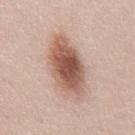<tbp_lesion>
  <biopsy_status>not biopsied; imaged during a skin examination</biopsy_status>
  <patient>
    <sex>male</sex>
    <age_approx>50</age_approx>
  </patient>
  <site>abdomen</site>
  <lesion_size>
    <long_diameter_mm_approx>8.5</long_diameter_mm_approx>
  </lesion_size>
  <image>
    <source>total-body photography crop</source>
    <field_of_view_mm>15</field_of_view_mm>
  </image>
  <lighting>white-light</lighting>
  <automated_metrics>
    <vs_skin_darker_L>16.0</vs_skin_darker_L>
    <vs_skin_contrast_norm>10.0</vs_skin_contrast_norm>
    <border_irregularity_0_10>2.5</border_irregularity_0_10>
    <color_variation_0_10>6.5</color_variation_0_10>
    <peripheral_color_asymmetry>1.5</peripheral_color_asymmetry>
    <nevus_likeness_0_100>100</nevus_likeness_0_100>
  </automated_metrics>
</tbp_lesion>